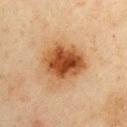  lesion_size:
    long_diameter_mm_approx: 5.5
  image:
    source: total-body photography crop
    field_of_view_mm: 15
  patient:
    sex: male
    age_approx: 45
  lighting: cross-polarized
  site: right upper arm
  automated_metrics:
    area_mm2_approx: 20.0
    cielab_L: 42
    cielab_a: 21
    cielab_b: 34
    vs_skin_darker_L: 13.0
    vs_skin_contrast_norm: 10.5
    nevus_likeness_0_100: 100
    lesion_detection_confidence_0_100: 100